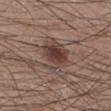This lesion was catalogued during total-body skin photography and was not selected for biopsy. A roughly 15 mm field-of-view crop from a total-body skin photograph. Located on the leg. The subject is a male aged approximately 20. About 4 mm across. An algorithmic analysis of the crop reported a footprint of about 12 mm² and two-axis asymmetry of about 0.25. The analysis additionally found a classifier nevus-likeness of about 100/100 and a lesion-detection confidence of about 100/100.